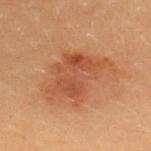Part of a total-body skin-imaging series; this lesion was reviewed on a skin check and was not flagged for biopsy. The subject is a female aged approximately 20. A 15 mm crop from a total-body photograph taken for skin-cancer surveillance. The lesion is on the upper back.